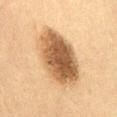Q: Was this lesion biopsied?
A: catalogued during a skin exam; not biopsied